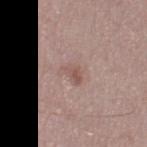Part of a total-body skin-imaging series; this lesion was reviewed on a skin check and was not flagged for biopsy. A male patient roughly 70 years of age. This is a white-light tile. A 15 mm crop from a total-body photograph taken for skin-cancer surveillance. From the left thigh.